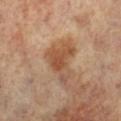Recorded during total-body skin imaging; not selected for excision or biopsy. A female subject about 65 years old. A 15 mm close-up tile from a total-body photography series done for melanoma screening. The lesion is located on the left leg.